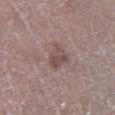{
  "biopsy_status": "not biopsied; imaged during a skin examination",
  "site": "left lower leg",
  "lighting": "white-light",
  "image": {
    "source": "total-body photography crop",
    "field_of_view_mm": 15
  },
  "patient": {
    "sex": "male",
    "age_approx": 70
  },
  "lesion_size": {
    "long_diameter_mm_approx": 3.5
  },
  "automated_metrics": {
    "cielab_L": 48,
    "cielab_a": 17,
    "cielab_b": 19,
    "vs_skin_darker_L": 9.0,
    "vs_skin_contrast_norm": 6.5,
    "color_variation_0_10": 3.5,
    "peripheral_color_asymmetry": 1.0
  }
}